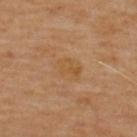<record>
<biopsy_status>not biopsied; imaged during a skin examination</biopsy_status>
<site>upper back</site>
<image>
  <source>total-body photography crop</source>
  <field_of_view_mm>15</field_of_view_mm>
</image>
<patient>
  <sex>male</sex>
  <age_approx>70</age_approx>
</patient>
<lighting>cross-polarized</lighting>
</record>